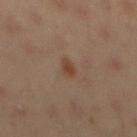Imaged during a routine full-body skin examination; the lesion was not biopsied and no histopathology is available.
The lesion is on the back.
A male subject, in their mid- to late 40s.
The recorded lesion diameter is about 2.5 mm.
A region of skin cropped from a whole-body photographic capture, roughly 15 mm wide.